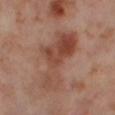Captured during whole-body skin photography for melanoma surveillance; the lesion was not biopsied.
An algorithmic analysis of the crop reported an average lesion color of about L≈47 a*≈23 b*≈28 (CIELAB), roughly 9 lightness units darker than nearby skin, and a normalized border contrast of about 7.
Measured at roughly 9 mm in maximum diameter.
On the leg.
A lesion tile, about 15 mm wide, cut from a 3D total-body photograph.
A female subject aged 53 to 57.